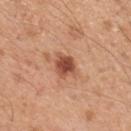Q: Is there a histopathology result?
A: catalogued during a skin exam; not biopsied
Q: What is the anatomic site?
A: the back
Q: Who is the patient?
A: male, aged 43–47
Q: How was the tile lit?
A: white-light
Q: How was this image acquired?
A: ~15 mm crop, total-body skin-cancer survey